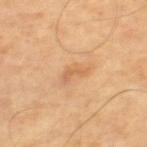workup = catalogued during a skin exam; not biopsied | subject = male, aged approximately 65 | image source = ~15 mm tile from a whole-body skin photo | diameter = about 3 mm | illumination = cross-polarized illumination.